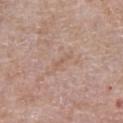workup: imaged on a skin check; not biopsied
subject: male, aged approximately 80
illumination: white-light
lesion size: about 2.5 mm
site: the chest
imaging modality: 15 mm crop, total-body photography